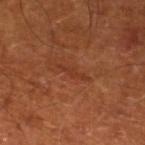Case summary:
* follow-up: no biopsy performed (imaged during a skin exam)
* size: ≈3 mm
* anatomic site: the left lower leg
* patient: in their mid-60s
* image source: 15 mm crop, total-body photography
* lighting: cross-polarized